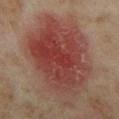The lesion was photographed on a routine skin check and not biopsied; there is no pathology result. A female patient, aged approximately 35. A close-up tile cropped from a whole-body skin photograph, about 15 mm across. Located on the left thigh. Captured under cross-polarized illumination. An algorithmic analysis of the crop reported an area of roughly 65 mm² and a symmetry-axis asymmetry near 0.2. It also reported a mean CIELAB color near L≈40 a*≈24 b*≈24, roughly 10 lightness units darker than nearby skin, and a normalized border contrast of about 8.5. And it measured a border-irregularity rating of about 3/10, internal color variation of about 8.5 on a 0–10 scale, and a peripheral color-asymmetry measure near 2.5. And it measured an automated nevus-likeness rating near 100 out of 100.